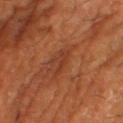biopsy status: total-body-photography surveillance lesion; no biopsy | site: the leg | lesion size: ~3 mm (longest diameter) | subject: male, roughly 60 years of age | image: total-body-photography crop, ~15 mm field of view | automated metrics: a mean CIELAB color near L≈36 a*≈26 b*≈32 and a lesion-to-skin contrast of about 5 (normalized; higher = more distinct) | illumination: cross-polarized.